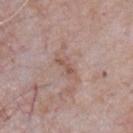follow-up = total-body-photography surveillance lesion; no biopsy | imaging modality = ~15 mm tile from a whole-body skin photo | anatomic site = the chest | tile lighting = white-light illumination | subject = male, in their mid- to late 60s | TBP lesion metrics = a lesion area of about 4.5 mm², a shape eccentricity near 0.9, and a symmetry-axis asymmetry near 0.35; a lesion color around L≈55 a*≈18 b*≈24 in CIELAB and roughly 7 lightness units darker than nearby skin; a nevus-likeness score of about 0/100 and a lesion-detection confidence of about 95/100 | diameter = ≈3.5 mm.